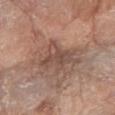Clinical impression: Captured during whole-body skin photography for melanoma surveillance; the lesion was not biopsied. Acquisition and patient details: The lesion is on the right forearm. Automated tile analysis of the lesion measured roughly 8 lightness units darker than nearby skin and a normalized lesion–skin contrast near 6.5. The software also gave peripheral color asymmetry of about 1.5. A roughly 15 mm field-of-view crop from a total-body skin photograph. Approximately 5 mm at its widest. A female patient, aged 73–77.